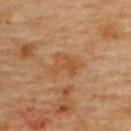Background:
The tile uses cross-polarized illumination. Automated image analysis of the tile measured a footprint of about 7 mm², a shape eccentricity near 0.65, and a shape-asymmetry score of about 0.35 (0 = symmetric). It also reported border irregularity of about 4.5 on a 0–10 scale, a color-variation rating of about 3.5/10, and a peripheral color-asymmetry measure near 1. The software also gave a classifier nevus-likeness of about 0/100 and a lesion-detection confidence of about 100/100. The lesion's longest dimension is about 3.5 mm. A roughly 15 mm field-of-view crop from a total-body skin photograph. A female subject aged approximately 60. From the upper back.